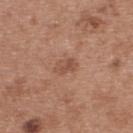Assessment:
Captured during whole-body skin photography for melanoma surveillance; the lesion was not biopsied.
Acquisition and patient details:
A region of skin cropped from a whole-body photographic capture, roughly 15 mm wide. Imaged with white-light lighting. On the upper back. A female patient, aged 38–42. An algorithmic analysis of the crop reported a border-irregularity index near 3/10, a within-lesion color-variation index near 3.5/10, and peripheral color asymmetry of about 1.5.